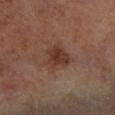Q: Was this lesion biopsied?
A: catalogued during a skin exam; not biopsied
Q: What are the patient's age and sex?
A: male, roughly 60 years of age
Q: What is the imaging modality?
A: 15 mm crop, total-body photography
Q: Lesion location?
A: the right lower leg